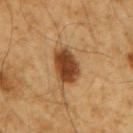lesion size=about 4.5 mm | automated lesion analysis=a footprint of about 10 mm² and two-axis asymmetry of about 0.15; an average lesion color of about L≈39 a*≈20 b*≈34 (CIELAB) and a lesion–skin lightness drop of about 15; border irregularity of about 2 on a 0–10 scale, a within-lesion color-variation index near 4/10, and a peripheral color-asymmetry measure near 1; an automated nevus-likeness rating near 95 out of 100 and a detector confidence of about 100 out of 100 that the crop contains a lesion | patient=male, roughly 60 years of age | image=15 mm crop, total-body photography | anatomic site=the right upper arm | illumination=cross-polarized illumination.